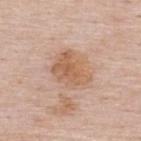Case summary:
– workup · no biopsy performed (imaged during a skin exam)
– subject · female, aged approximately 50
– acquisition · ~15 mm tile from a whole-body skin photo
– site · the upper back
– TBP lesion metrics · a lesion-to-skin contrast of about 7 (normalized; higher = more distinct); a border-irregularity rating of about 3/10 and a within-lesion color-variation index near 3.5/10; a classifier nevus-likeness of about 5/100
– lesion diameter · ≈4.5 mm